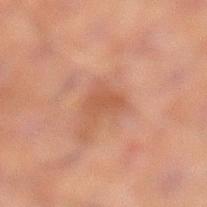Notes:
* follow-up · imaged on a skin check; not biopsied
* imaging modality · 15 mm crop, total-body photography
* diameter · ~3 mm (longest diameter)
* anatomic site · the left lower leg
* TBP lesion metrics · an area of roughly 5.5 mm², a shape eccentricity near 0.7, and a symmetry-axis asymmetry near 0.35; border irregularity of about 3.5 on a 0–10 scale, a color-variation rating of about 1.5/10, and peripheral color asymmetry of about 0.5
* patient · male, aged approximately 60
* tile lighting · cross-polarized